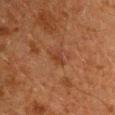Captured during whole-body skin photography for melanoma surveillance; the lesion was not biopsied.
Located on the left upper arm.
A male patient aged 58 to 62.
A close-up tile cropped from a whole-body skin photograph, about 15 mm across.